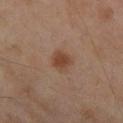Imaged during a routine full-body skin examination; the lesion was not biopsied and no histopathology is available.
Imaged with cross-polarized lighting.
A lesion tile, about 15 mm wide, cut from a 3D total-body photograph.
The patient is a female in their 60s.
Longest diameter approximately 2.5 mm.
The lesion is on the leg.
The total-body-photography lesion software estimated a border-irregularity rating of about 1.5/10. And it measured a nevus-likeness score of about 95/100 and a lesion-detection confidence of about 100/100.